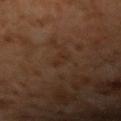workup=no biopsy performed (imaged during a skin exam) | lighting=cross-polarized | lesion size=≈3 mm | subject=male, aged 58–62 | image=15 mm crop, total-body photography | anatomic site=the arm.